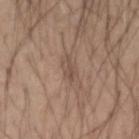Assessment: This lesion was catalogued during total-body skin photography and was not selected for biopsy. Image and clinical context: The lesion is located on the left forearm. About 2.5 mm across. A region of skin cropped from a whole-body photographic capture, roughly 15 mm wide. A male patient aged 53 to 57. Captured under white-light illumination. Automated tile analysis of the lesion measured a lesion area of about 2.5 mm², an eccentricity of roughly 0.9, and a shape-asymmetry score of about 0.5 (0 = symmetric). And it measured a border-irregularity rating of about 5.5/10, a within-lesion color-variation index near 0/10, and radial color variation of about 0. And it measured a classifier nevus-likeness of about 0/100 and a detector confidence of about 75 out of 100 that the crop contains a lesion.